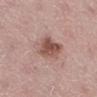follow-up: imaged on a skin check; not biopsied | image-analysis metrics: a lesion area of about 15 mm², an eccentricity of roughly 0.85, and a symmetry-axis asymmetry near 0.25; a mean CIELAB color near L≈55 a*≈19 b*≈24, a lesion–skin lightness drop of about 11, and a normalized border contrast of about 7.5; a border-irregularity rating of about 3/10, a within-lesion color-variation index near 8/10, and a peripheral color-asymmetry measure near 3; an automated nevus-likeness rating near 55 out of 100 and a detector confidence of about 100 out of 100 that the crop contains a lesion | site: the left lower leg | size: about 6 mm | illumination: white-light illumination | image: ~15 mm tile from a whole-body skin photo | patient: female, about 45 years old.